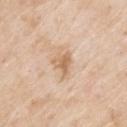This lesion was catalogued during total-body skin photography and was not selected for biopsy.